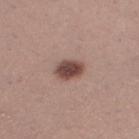The lesion is located on the leg. Captured under white-light illumination. The lesion's longest dimension is about 3.5 mm. The patient is a female about 30 years old. The lesion-visualizer software estimated a lesion color around L≈46 a*≈19 b*≈22 in CIELAB, about 15 CIELAB-L* units darker than the surrounding skin, and a normalized lesion–skin contrast near 11. The software also gave a border-irregularity rating of about 1.5/10, a within-lesion color-variation index near 3.5/10, and peripheral color asymmetry of about 1. This image is a 15 mm lesion crop taken from a total-body photograph.